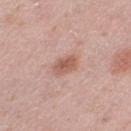workup: catalogued during a skin exam; not biopsied | imaging modality: 15 mm crop, total-body photography | image-analysis metrics: a lesion area of about 6 mm², an outline eccentricity of about 0.75 (0 = round, 1 = elongated), and a symmetry-axis asymmetry near 0.1; a border-irregularity index near 1.5/10, a color-variation rating of about 3/10, and a peripheral color-asymmetry measure near 1; an automated nevus-likeness rating near 85 out of 100 | body site: the left thigh | lighting: white-light | subject: female, roughly 40 years of age.